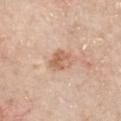Clinical impression: The lesion was tiled from a total-body skin photograph and was not biopsied. Clinical summary: From the chest. The patient is a male about 65 years old. This image is a 15 mm lesion crop taken from a total-body photograph.